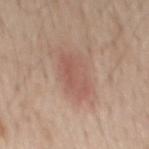No biopsy was performed on this lesion — it was imaged during a full skin examination and was not determined to be concerning.
Imaged with white-light lighting.
A 15 mm close-up extracted from a 3D total-body photography capture.
From the mid back.
A male patient aged 58–62.